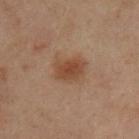{"biopsy_status": "not biopsied; imaged during a skin examination", "automated_metrics": {"border_irregularity_0_10": 2.5, "color_variation_0_10": 2.0, "peripheral_color_asymmetry": 0.5, "nevus_likeness_0_100": 80, "lesion_detection_confidence_0_100": 100}, "patient": {"sex": "male", "age_approx": 55}, "site": "chest", "lesion_size": {"long_diameter_mm_approx": 3.5}, "image": {"source": "total-body photography crop", "field_of_view_mm": 15}}